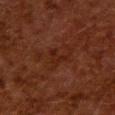Imaged during a routine full-body skin examination; the lesion was not biopsied and no histopathology is available. The subject is a female in their 50s. A lesion tile, about 15 mm wide, cut from a 3D total-body photograph. Located on the upper back. Imaged with cross-polarized lighting. The lesion-visualizer software estimated an eccentricity of roughly 0.7 and a shape-asymmetry score of about 0.65 (0 = symmetric). The analysis additionally found a mean CIELAB color near L≈19 a*≈20 b*≈23, a lesion–skin lightness drop of about 4, and a normalized lesion–skin contrast near 5.